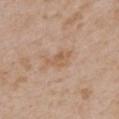Impression: This lesion was catalogued during total-body skin photography and was not selected for biopsy. Acquisition and patient details: Cropped from a total-body skin-imaging series; the visible field is about 15 mm. The lesion is on the upper back. The subject is a female aged 28 to 32.